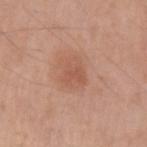Captured during whole-body skin photography for melanoma surveillance; the lesion was not biopsied. A close-up tile cropped from a whole-body skin photograph, about 15 mm across. A male patient, approximately 60 years of age. The lesion is located on the left upper arm.